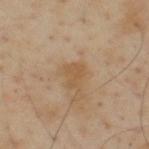Clinical impression: Part of a total-body skin-imaging series; this lesion was reviewed on a skin check and was not flagged for biopsy. Acquisition and patient details: The patient is a male in their mid- to late 50s. An algorithmic analysis of the crop reported an area of roughly 4.5 mm² and a symmetry-axis asymmetry near 0.4. And it measured a mean CIELAB color near L≈54 a*≈17 b*≈35, about 6 CIELAB-L* units darker than the surrounding skin, and a normalized border contrast of about 6. It also reported a border-irregularity index near 3.5/10 and peripheral color asymmetry of about 0.5. About 2.5 mm across. The tile uses cross-polarized illumination. A 15 mm crop from a total-body photograph taken for skin-cancer surveillance. The lesion is on the upper back.